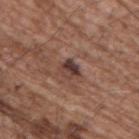Case summary:
– workup — imaged on a skin check; not biopsied
– automated lesion analysis — a lesion area of about 4.5 mm², an eccentricity of roughly 0.6, and a symmetry-axis asymmetry near 0.2; a lesion–skin lightness drop of about 11 and a normalized lesion–skin contrast near 10; a lesion-detection confidence of about 100/100
– illumination — white-light illumination
– imaging modality — ~15 mm crop, total-body skin-cancer survey
– site — the upper back
– subject — male, aged around 70